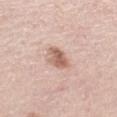| key | value |
|---|---|
| workup | total-body-photography surveillance lesion; no biopsy |
| subject | female, in their mid-60s |
| size | ≈3 mm |
| tile lighting | white-light illumination |
| imaging modality | ~15 mm tile from a whole-body skin photo |
| automated metrics | a border-irregularity rating of about 2.5/10 and radial color variation of about 1; a nevus-likeness score of about 85/100 |
| body site | the leg |